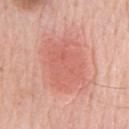Assessment: This lesion was catalogued during total-body skin photography and was not selected for biopsy. Clinical summary: The total-body-photography lesion software estimated a footprint of about 31 mm², an outline eccentricity of about 0.7 (0 = round, 1 = elongated), and a symmetry-axis asymmetry near 0.15. The analysis additionally found an average lesion color of about L≈63 a*≈28 b*≈29 (CIELAB), about 8 CIELAB-L* units darker than the surrounding skin, and a normalized border contrast of about 5. The analysis additionally found internal color variation of about 3 on a 0–10 scale and radial color variation of about 1. The software also gave an automated nevus-likeness rating near 90 out of 100 and a detector confidence of about 100 out of 100 that the crop contains a lesion. A male patient aged 78–82. Imaged with white-light lighting. Located on the chest. This image is a 15 mm lesion crop taken from a total-body photograph.